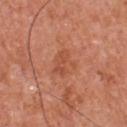| feature | finding |
|---|---|
| biopsy status | imaged on a skin check; not biopsied |
| location | the chest |
| automated metrics | border irregularity of about 4.5 on a 0–10 scale and a peripheral color-asymmetry measure near 0.5; an automated nevus-likeness rating near 10 out of 100 and a detector confidence of about 100 out of 100 that the crop contains a lesion |
| subject | male, approximately 55 years of age |
| acquisition | 15 mm crop, total-body photography |
| size | about 3 mm |